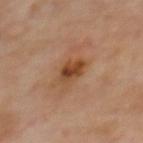This lesion was catalogued during total-body skin photography and was not selected for biopsy. A female patient about 50 years old. Located on the upper back. Cropped from a total-body skin-imaging series; the visible field is about 15 mm. Automated image analysis of the tile measured a lesion color around L≈46 a*≈21 b*≈35 in CIELAB and a normalized border contrast of about 8. And it measured internal color variation of about 6.5 on a 0–10 scale and radial color variation of about 2. The tile uses cross-polarized illumination.